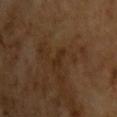Case summary:
– workup: imaged on a skin check; not biopsied
– subject: male, in their mid-60s
– image source: ~15 mm crop, total-body skin-cancer survey